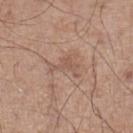Clinical impression: The lesion was tiled from a total-body skin photograph and was not biopsied. Clinical summary: The lesion is on the left lower leg. The tile uses white-light illumination. Cropped from a whole-body photographic skin survey; the tile spans about 15 mm. A male patient aged 58 to 62.